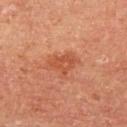Notes:
* notes — catalogued during a skin exam; not biopsied
* TBP lesion metrics — a lesion area of about 7.5 mm², an outline eccentricity of about 0.75 (0 = round, 1 = elongated), and two-axis asymmetry of about 0.4; a border-irregularity index near 4/10, a color-variation rating of about 3.5/10, and a peripheral color-asymmetry measure near 1.5; a classifier nevus-likeness of about 10/100 and lesion-presence confidence of about 100/100
* patient — male, in their mid-60s
* image — ~15 mm tile from a whole-body skin photo
* body site — the arm
* illumination — cross-polarized illumination